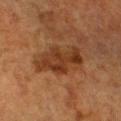Impression:
The lesion was photographed on a routine skin check and not biopsied; there is no pathology result.
Image and clinical context:
A region of skin cropped from a whole-body photographic capture, roughly 15 mm wide. This is a cross-polarized tile. The subject is a female roughly 55 years of age. The total-body-photography lesion software estimated a shape eccentricity near 0.85. The software also gave a mean CIELAB color near L≈30 a*≈20 b*≈29, about 8 CIELAB-L* units darker than the surrounding skin, and a normalized border contrast of about 8.5. The analysis additionally found a within-lesion color-variation index near 4/10 and peripheral color asymmetry of about 1.5. The software also gave a classifier nevus-likeness of about 10/100 and a detector confidence of about 100 out of 100 that the crop contains a lesion. The lesion is located on the head or neck.